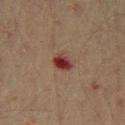Q: What kind of image is this?
A: 15 mm crop, total-body photography
Q: What is the anatomic site?
A: the left thigh
Q: Automated lesion metrics?
A: border irregularity of about 1.5 on a 0–10 scale, internal color variation of about 5 on a 0–10 scale, and a peripheral color-asymmetry measure near 1.5; a nevus-likeness score of about 0/100 and a lesion-detection confidence of about 100/100
Q: Illumination type?
A: cross-polarized
Q: Patient demographics?
A: male, aged approximately 45
Q: Lesion size?
A: ~2.5 mm (longest diameter)